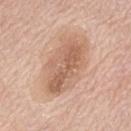Assessment:
This lesion was catalogued during total-body skin photography and was not selected for biopsy.
Context:
Located on the abdomen. A female subject aged 73–77. Captured under white-light illumination. The recorded lesion diameter is about 7.5 mm. Cropped from a total-body skin-imaging series; the visible field is about 15 mm.